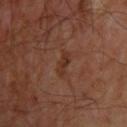<tbp_lesion>
<biopsy_status>not biopsied; imaged during a skin examination</biopsy_status>
<patient>
  <sex>male</sex>
  <age_approx>55</age_approx>
</patient>
<site>upper back</site>
<lighting>cross-polarized</lighting>
<automated_metrics>
  <cielab_L>32</cielab_L>
  <cielab_a>21</cielab_a>
  <cielab_b>27</cielab_b>
  <vs_skin_darker_L>6.0</vs_skin_darker_L>
</automated_metrics>
<lesion_size>
  <long_diameter_mm_approx>3.0</long_diameter_mm_approx>
</lesion_size>
<image>
  <source>total-body photography crop</source>
  <field_of_view_mm>15</field_of_view_mm>
</image>
</tbp_lesion>